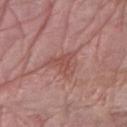Impression:
No biopsy was performed on this lesion — it was imaged during a full skin examination and was not determined to be concerning.
Clinical summary:
Approximately 4 mm at its widest. This image is a 15 mm lesion crop taken from a total-body photograph. A female patient, aged approximately 65. An algorithmic analysis of the crop reported a footprint of about 7.5 mm², a shape eccentricity near 0.25, and a shape-asymmetry score of about 0.5 (0 = symmetric). It also reported a lesion color around L≈51 a*≈24 b*≈24 in CIELAB, a lesion–skin lightness drop of about 7, and a normalized lesion–skin contrast near 5.5. It also reported a border-irregularity rating of about 6/10 and peripheral color asymmetry of about 0.5. The lesion is on the right forearm.